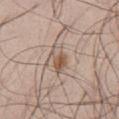| key | value |
|---|---|
| notes | total-body-photography surveillance lesion; no biopsy |
| automated lesion analysis | a footprint of about 5.5 mm², a shape eccentricity near 0.8, and a shape-asymmetry score of about 0.3 (0 = symmetric); a peripheral color-asymmetry measure near 3 |
| location | the front of the torso |
| imaging modality | ~15 mm tile from a whole-body skin photo |
| subject | male, aged 48 to 52 |
| lesion size | ~3.5 mm (longest diameter) |